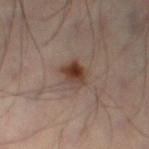workup: catalogued during a skin exam; not biopsied | patient: male, approximately 50 years of age | illumination: cross-polarized | size: ≈3 mm | image source: ~15 mm crop, total-body skin-cancer survey | image-analysis metrics: an area of roughly 6.5 mm², a shape eccentricity near 0.05, and a symmetry-axis asymmetry near 0.2; about 11 CIELAB-L* units darker than the surrounding skin and a normalized lesion–skin contrast near 9.5; border irregularity of about 2 on a 0–10 scale, a color-variation rating of about 8/10, and radial color variation of about 3; a classifier nevus-likeness of about 95/100 and lesion-presence confidence of about 100/100 | location: the lower back.